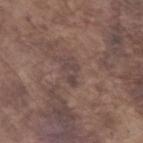follow-up — total-body-photography surveillance lesion; no biopsy | automated metrics — a classifier nevus-likeness of about 0/100 and a detector confidence of about 70 out of 100 that the crop contains a lesion | image source — ~15 mm tile from a whole-body skin photo | patient — male, roughly 75 years of age | lesion size — about 3 mm | lighting — white-light | location — the left forearm.